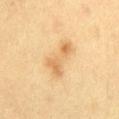Imaged during a routine full-body skin examination; the lesion was not biopsied and no histopathology is available. The lesion's longest dimension is about 5.5 mm. Captured under cross-polarized illumination. The patient is a female approximately 30 years of age. From the abdomen. A close-up tile cropped from a whole-body skin photograph, about 15 mm across.